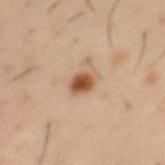From the mid back. The recorded lesion diameter is about 3 mm. A roughly 15 mm field-of-view crop from a total-body skin photograph. A male patient, in their mid- to late 50s. The tile uses cross-polarized illumination.